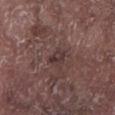Notes:
– notes — imaged on a skin check; not biopsied
– imaging modality — total-body-photography crop, ~15 mm field of view
– anatomic site — the left lower leg
– lesion diameter — ~3 mm (longest diameter)
– lighting — white-light illumination
– subject — male, aged approximately 75